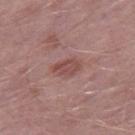follow-up — no biopsy performed (imaged during a skin exam) | lesion size — about 3.5 mm | anatomic site — the right thigh | subject — male, about 50 years old | image source — total-body-photography crop, ~15 mm field of view | lighting — white-light illumination.